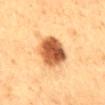Recorded during total-body skin imaging; not selected for excision or biopsy.
The lesion is on the mid back.
A male subject aged 58–62.
A roughly 15 mm field-of-view crop from a total-body skin photograph.